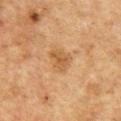Part of a total-body skin-imaging series; this lesion was reviewed on a skin check and was not flagged for biopsy.
The lesion is located on the chest.
A male subject in their mid-70s.
The total-body-photography lesion software estimated a footprint of about 7 mm² and a shape-asymmetry score of about 0.25 (0 = symmetric). The analysis additionally found a mean CIELAB color near L≈46 a*≈17 b*≈33, about 7 CIELAB-L* units darker than the surrounding skin, and a lesion-to-skin contrast of about 6 (normalized; higher = more distinct).
Approximately 3 mm at its widest.
This image is a 15 mm lesion crop taken from a total-body photograph.
Imaged with cross-polarized lighting.